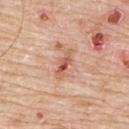Q: Was a biopsy performed?
A: no biopsy performed (imaged during a skin exam)
Q: Illumination type?
A: white-light
Q: How was this image acquired?
A: ~15 mm tile from a whole-body skin photo
Q: Where on the body is the lesion?
A: the upper back
Q: Patient demographics?
A: male, aged 63–67
Q: What did automated image analysis measure?
A: an area of roughly 5 mm², an outline eccentricity of about 0.9 (0 = round, 1 = elongated), and a symmetry-axis asymmetry near 0.35; a lesion color around L≈59 a*≈25 b*≈31 in CIELAB and a normalized border contrast of about 7
Q: How large is the lesion?
A: ~4 mm (longest diameter)